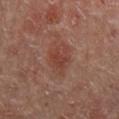biopsy status = catalogued during a skin exam; not biopsied
image source = ~15 mm crop, total-body skin-cancer survey
patient = male, in their mid- to late 60s
lesion diameter = about 4.5 mm
anatomic site = the mid back